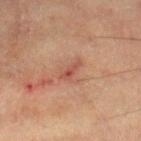Image and clinical context:
Automated image analysis of the tile measured a border-irregularity rating of about 4.5/10, internal color variation of about 0 on a 0–10 scale, and peripheral color asymmetry of about 0. The analysis additionally found a nevus-likeness score of about 0/100. The lesion's longest dimension is about 3 mm. A 15 mm close-up tile from a total-body photography series done for melanoma screening. The tile uses cross-polarized illumination. A male patient aged approximately 55. Located on the left leg.